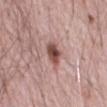acquisition — total-body-photography crop, ~15 mm field of view
diameter — about 3.5 mm
patient — male, aged 68 to 72
anatomic site — the abdomen
illumination — white-light illumination